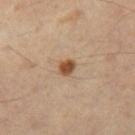Background:
Cropped from a whole-body photographic skin survey; the tile spans about 15 mm. The lesion is on the right thigh. The recorded lesion diameter is about 2 mm. A male patient aged 53 to 57.